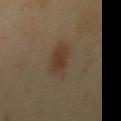Recorded during total-body skin imaging; not selected for excision or biopsy. A male subject, aged approximately 40. Located on the right upper arm. A roughly 15 mm field-of-view crop from a total-body skin photograph. This is a cross-polarized tile. The total-body-photography lesion software estimated a mean CIELAB color near L≈36 a*≈15 b*≈26, about 7 CIELAB-L* units darker than the surrounding skin, and a normalized border contrast of about 7. The analysis additionally found a border-irregularity index near 2/10. Measured at roughly 3.5 mm in maximum diameter.